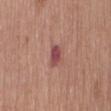Longest diameter approximately 2.5 mm.
Automated tile analysis of the lesion measured a lesion area of about 4 mm² and an eccentricity of roughly 0.8. It also reported a mean CIELAB color near L≈48 a*≈27 b*≈21 and roughly 11 lightness units darker than nearby skin. It also reported a color-variation rating of about 2.5/10 and a peripheral color-asymmetry measure near 0.5.
From the mid back.
A female subject, aged 53–57.
A region of skin cropped from a whole-body photographic capture, roughly 15 mm wide.